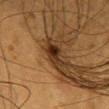The lesion was tiled from a total-body skin photograph and was not biopsied.
This image is a 15 mm lesion crop taken from a total-body photograph.
The lesion-visualizer software estimated an eccentricity of roughly 0.9. And it measured an average lesion color of about L≈27 a*≈17 b*≈28 (CIELAB) and a normalized lesion–skin contrast near 12.5.
The subject is a female aged around 40.
The tile uses cross-polarized illumination.
Approximately 4 mm at its widest.
On the head or neck.